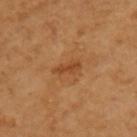Clinical impression:
The lesion was tiled from a total-body skin photograph and was not biopsied.
Clinical summary:
A 15 mm crop from a total-body photograph taken for skin-cancer surveillance. A female patient aged around 55. The lesion is located on the arm.